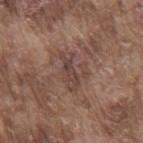{"biopsy_status": "not biopsied; imaged during a skin examination", "patient": {"sex": "male", "age_approx": 75}, "image": {"source": "total-body photography crop", "field_of_view_mm": 15}, "site": "mid back"}